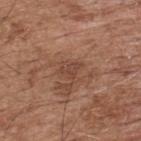<tbp_lesion>
  <biopsy_status>not biopsied; imaged during a skin examination</biopsy_status>
  <site>back</site>
  <automated_metrics>
    <cielab_L>44</cielab_L>
    <cielab_a>21</cielab_a>
    <cielab_b>28</cielab_b>
    <vs_skin_darker_L>7.0</vs_skin_darker_L>
    <border_irregularity_0_10>5.0</border_irregularity_0_10>
    <color_variation_0_10>2.0</color_variation_0_10>
    <peripheral_color_asymmetry>0.5</peripheral_color_asymmetry>
    <nevus_likeness_0_100>0</nevus_likeness_0_100>
    <lesion_detection_confidence_0_100>100</lesion_detection_confidence_0_100>
  </automated_metrics>
  <patient>
    <sex>male</sex>
    <age_approx>75</age_approx>
  </patient>
  <image>
    <source>total-body photography crop</source>
    <field_of_view_mm>15</field_of_view_mm>
  </image>
  <lesion_size>
    <long_diameter_mm_approx>3.0</long_diameter_mm_approx>
  </lesion_size>
</tbp_lesion>